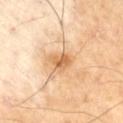| feature | finding |
|---|---|
| location | the right thigh |
| image source | ~15 mm tile from a whole-body skin photo |
| patient | male, approximately 70 years of age |